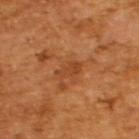Q: What are the patient's age and sex?
A: male, aged 63–67
Q: Automated lesion metrics?
A: a nevus-likeness score of about 0/100 and a detector confidence of about 100 out of 100 that the crop contains a lesion
Q: How was this image acquired?
A: total-body-photography crop, ~15 mm field of view
Q: What lighting was used for the tile?
A: cross-polarized illumination
Q: Lesion size?
A: about 3 mm